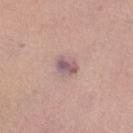| field | value |
|---|---|
| workup | total-body-photography surveillance lesion; no biopsy |
| acquisition | ~15 mm crop, total-body skin-cancer survey |
| illumination | white-light |
| automated lesion analysis | a footprint of about 4.5 mm², an outline eccentricity of about 0.7 (0 = round, 1 = elongated), and two-axis asymmetry of about 0.25; a mean CIELAB color near L≈56 a*≈18 b*≈17 and a normalized lesion–skin contrast near 8.5 |
| body site | the left lower leg |
| patient | female, approximately 35 years of age |
| lesion size | ~2.5 mm (longest diameter) |